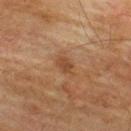Assessment: Imaged during a routine full-body skin examination; the lesion was not biopsied and no histopathology is available. Background: The total-body-photography lesion software estimated an average lesion color of about L≈38 a*≈18 b*≈29 (CIELAB), roughly 6 lightness units darker than nearby skin, and a lesion-to-skin contrast of about 6 (normalized; higher = more distinct). A male subject, roughly 75 years of age. The recorded lesion diameter is about 3 mm. Cropped from a whole-body photographic skin survey; the tile spans about 15 mm. The lesion is located on the back.